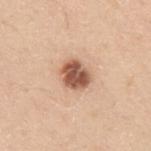Recorded during total-body skin imaging; not selected for excision or biopsy.
A region of skin cropped from a whole-body photographic capture, roughly 15 mm wide.
The tile uses white-light illumination.
A male patient about 30 years old.
On the left upper arm.
The lesion's longest dimension is about 3.5 mm.